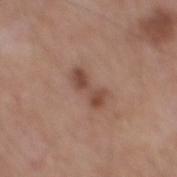This image is a 15 mm lesion crop taken from a total-body photograph.
A male patient in their mid-50s.
The lesion's longest dimension is about 4 mm.
From the mid back.